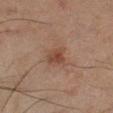On the leg.
This is a cross-polarized tile.
Automated tile analysis of the lesion measured a lesion area of about 5 mm² and two-axis asymmetry of about 0.3. The analysis additionally found a classifier nevus-likeness of about 30/100 and a lesion-detection confidence of about 100/100.
Cropped from a total-body skin-imaging series; the visible field is about 15 mm.
A male patient, in their mid- to late 60s.